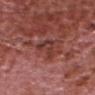Part of a total-body skin-imaging series; this lesion was reviewed on a skin check and was not flagged for biopsy. A male subject aged 73–77. The lesion-visualizer software estimated an eccentricity of roughly 0.85 and a shape-asymmetry score of about 0.55 (0 = symmetric). The software also gave a classifier nevus-likeness of about 0/100 and lesion-presence confidence of about 90/100. The lesion is on the head or neck. The lesion's longest dimension is about 4 mm. Cropped from a total-body skin-imaging series; the visible field is about 15 mm.